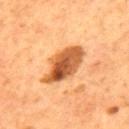Q: Was this lesion biopsied?
A: total-body-photography surveillance lesion; no biopsy
Q: Lesion size?
A: ~6.5 mm (longest diameter)
Q: What lighting was used for the tile?
A: cross-polarized illumination
Q: Who is the patient?
A: male, in their mid- to late 50s
Q: What is the imaging modality?
A: 15 mm crop, total-body photography
Q: Lesion location?
A: the mid back